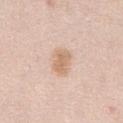This lesion was catalogued during total-body skin photography and was not selected for biopsy.